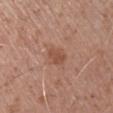Clinical impression:
No biopsy was performed on this lesion — it was imaged during a full skin examination and was not determined to be concerning.
Context:
The patient is a male aged 68–72. On the left upper arm. This image is a 15 mm lesion crop taken from a total-body photograph.